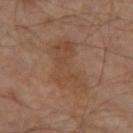  biopsy_status: not biopsied; imaged during a skin examination
  image:
    source: total-body photography crop
    field_of_view_mm: 15
  lighting: cross-polarized
  patient:
    sex: male
    age_approx: 65
  site: left thigh
  lesion_size:
    long_diameter_mm_approx: 7.0
  automated_metrics:
    cielab_L: 43
    cielab_a: 17
    cielab_b: 28
    vs_skin_darker_L: 5.0